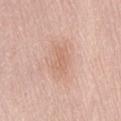Case summary:
* follow-up · catalogued during a skin exam; not biopsied
* body site · the lower back
* subject · female, aged 53–57
* image · ~15 mm crop, total-body skin-cancer survey
* diameter · about 3.5 mm
* TBP lesion metrics · a mean CIELAB color near L≈65 a*≈21 b*≈30, about 7 CIELAB-L* units darker than the surrounding skin, and a normalized lesion–skin contrast near 4.5